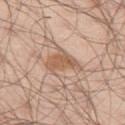image: ~15 mm crop, total-body skin-cancer survey | diameter: ~4.5 mm (longest diameter) | tile lighting: white-light illumination | image-analysis metrics: an area of roughly 8 mm², a shape eccentricity near 0.8, and a symmetry-axis asymmetry near 0.4; a within-lesion color-variation index near 2.5/10 and peripheral color asymmetry of about 1 | patient: male, aged 53–57 | location: the left thigh.